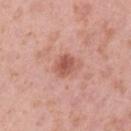biopsy status: catalogued during a skin exam; not biopsied | imaging modality: ~15 mm crop, total-body skin-cancer survey | subject: female, aged approximately 40 | image-analysis metrics: an area of roughly 4.5 mm² and an outline eccentricity of about 0.6 (0 = round, 1 = elongated); a mean CIELAB color near L≈55 a*≈27 b*≈28 and roughly 11 lightness units darker than nearby skin; a detector confidence of about 100 out of 100 that the crop contains a lesion | lighting: white-light illumination | size: ~2.5 mm (longest diameter) | site: the left upper arm.